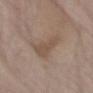workup: imaged on a skin check; not biopsied
imaging modality: ~15 mm tile from a whole-body skin photo
tile lighting: white-light illumination
lesion size: about 4 mm
patient: male, approximately 75 years of age
TBP lesion metrics: a lesion area of about 7.5 mm², an outline eccentricity of about 0.85 (0 = round, 1 = elongated), and a symmetry-axis asymmetry near 0.45; a classifier nevus-likeness of about 0/100 and a detector confidence of about 100 out of 100 that the crop contains a lesion
body site: the left forearm